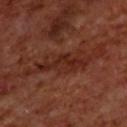size: ≈6 mm
patient: male, aged approximately 70
image source: ~15 mm crop, total-body skin-cancer survey
location: the upper back